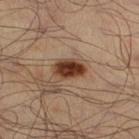Q: Was this lesion biopsied?
A: no biopsy performed (imaged during a skin exam)
Q: Lesion location?
A: the left leg
Q: Patient demographics?
A: male, aged 48–52
Q: How was this image acquired?
A: total-body-photography crop, ~15 mm field of view